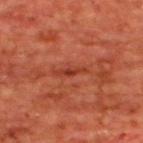follow-up = catalogued during a skin exam; not biopsied | image = ~15 mm tile from a whole-body skin photo | lighting = cross-polarized | size = about 2.5 mm | body site = the upper back | patient = male, aged around 65.